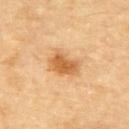{"biopsy_status": "not biopsied; imaged during a skin examination", "image": {"source": "total-body photography crop", "field_of_view_mm": 15}, "site": "back", "patient": {"sex": "male", "age_approx": "85+"}}